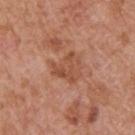Assessment:
Imaged during a routine full-body skin examination; the lesion was not biopsied and no histopathology is available.
Background:
Measured at roughly 4.5 mm in maximum diameter. On the upper back. A male patient aged 63–67. A roughly 15 mm field-of-view crop from a total-body skin photograph.